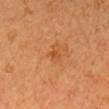Assessment: Captured during whole-body skin photography for melanoma surveillance; the lesion was not biopsied. Clinical summary: A 15 mm close-up extracted from a 3D total-body photography capture. Captured under cross-polarized illumination. The subject is a female approximately 55 years of age. The lesion's longest dimension is about 1 mm. On the head or neck.